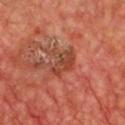Assessment: This lesion was catalogued during total-body skin photography and was not selected for biopsy. Clinical summary: A region of skin cropped from a whole-body photographic capture, roughly 15 mm wide. The lesion is on the chest. A male subject, about 65 years old.